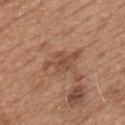Findings:
– workup: catalogued during a skin exam; not biopsied
– automated lesion analysis: an average lesion color of about L≈49 a*≈21 b*≈30 (CIELAB) and a lesion-to-skin contrast of about 6.5 (normalized; higher = more distinct); a color-variation rating of about 2.5/10
– diameter: about 4.5 mm
– lighting: white-light illumination
– image source: ~15 mm crop, total-body skin-cancer survey
– subject: male, in their mid-60s
– anatomic site: the chest